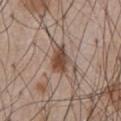Q: Is there a histopathology result?
A: imaged on a skin check; not biopsied
Q: Automated lesion metrics?
A: a nevus-likeness score of about 95/100 and a detector confidence of about 100 out of 100 that the crop contains a lesion
Q: What is the imaging modality?
A: total-body-photography crop, ~15 mm field of view
Q: Illumination type?
A: white-light
Q: Patient demographics?
A: male, aged approximately 60
Q: Where on the body is the lesion?
A: the chest
Q: What is the lesion's diameter?
A: ≈4 mm